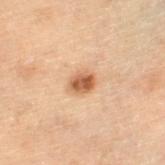Recorded during total-body skin imaging; not selected for excision or biopsy. The lesion is on the left thigh. Automated image analysis of the tile measured a lesion area of about 5 mm², an eccentricity of roughly 0.6, and a shape-asymmetry score of about 0.25 (0 = symmetric). The software also gave an average lesion color of about L≈44 a*≈18 b*≈29 (CIELAB), a lesion–skin lightness drop of about 12, and a normalized lesion–skin contrast near 9. The analysis additionally found a border-irregularity index near 2/10, a within-lesion color-variation index near 3.5/10, and a peripheral color-asymmetry measure near 1. The analysis additionally found a nevus-likeness score of about 95/100. A region of skin cropped from a whole-body photographic capture, roughly 15 mm wide. This is a cross-polarized tile. A male patient aged approximately 70. Longest diameter approximately 2.5 mm.